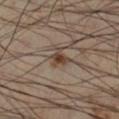{"biopsy_status": "not biopsied; imaged during a skin examination", "lesion_size": {"long_diameter_mm_approx": 2.0}, "lighting": "cross-polarized", "automated_metrics": {"area_mm2_approx": 3.0, "eccentricity": 0.7, "shape_asymmetry": 0.3, "vs_skin_darker_L": 11.0, "vs_skin_contrast_norm": 10.0, "border_irregularity_0_10": 2.5, "color_variation_0_10": 2.0, "peripheral_color_asymmetry": 0.5, "nevus_likeness_0_100": 90}, "patient": {"sex": "male", "age_approx": 40}, "image": {"source": "total-body photography crop", "field_of_view_mm": 15}, "site": "right lower leg"}